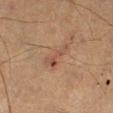The lesion was photographed on a routine skin check and not biopsied; there is no pathology result. A male patient aged around 60. Approximately 4 mm at its widest. The tile uses cross-polarized illumination. On the right lower leg. A 15 mm close-up tile from a total-body photography series done for melanoma screening.